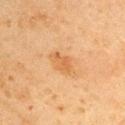Impression: This lesion was catalogued during total-body skin photography and was not selected for biopsy. Context: The tile uses cross-polarized illumination. A region of skin cropped from a whole-body photographic capture, roughly 15 mm wide. The lesion is located on the right upper arm. The patient is a male about 45 years old.